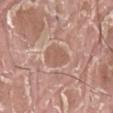Q: Was this lesion biopsied?
A: catalogued during a skin exam; not biopsied
Q: How was the tile lit?
A: white-light illumination
Q: Patient demographics?
A: male, roughly 40 years of age
Q: How was this image acquired?
A: 15 mm crop, total-body photography
Q: What is the anatomic site?
A: the left thigh